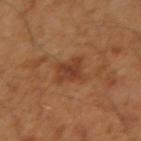anatomic site: the right upper arm
tile lighting: cross-polarized illumination
lesion diameter: ~3.5 mm (longest diameter)
automated lesion analysis: an area of roughly 7.5 mm² and a symmetry-axis asymmetry near 0.35; a mean CIELAB color near L≈40 a*≈23 b*≈32 and a normalized border contrast of about 7
subject: male, aged around 45
imaging modality: 15 mm crop, total-body photography